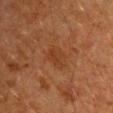Impression: This lesion was catalogued during total-body skin photography and was not selected for biopsy. Image and clinical context: This is a cross-polarized tile. Automated image analysis of the tile measured an outline eccentricity of about 0.75 (0 = round, 1 = elongated) and a symmetry-axis asymmetry near 0.2. The analysis additionally found a mean CIELAB color near L≈30 a*≈19 b*≈28, about 4 CIELAB-L* units darker than the surrounding skin, and a lesion-to-skin contrast of about 5 (normalized; higher = more distinct). The software also gave an automated nevus-likeness rating near 0 out of 100 and a lesion-detection confidence of about 100/100. A roughly 15 mm field-of-view crop from a total-body skin photograph. Longest diameter approximately 3.5 mm. The lesion is on the left upper arm. A male subject aged approximately 60.